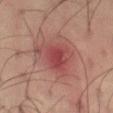Findings:
* workup: catalogued during a skin exam; not biopsied
* lesion size: ≈5.5 mm
* lighting: cross-polarized
* site: the left thigh
* subject: male, aged approximately 40
* acquisition: total-body-photography crop, ~15 mm field of view